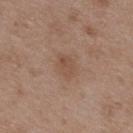Q: Is there a histopathology result?
A: no biopsy performed (imaged during a skin exam)
Q: How was this image acquired?
A: total-body-photography crop, ~15 mm field of view
Q: What is the anatomic site?
A: the upper back
Q: What are the patient's age and sex?
A: male, aged approximately 50